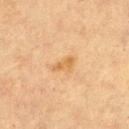Automated tile analysis of the lesion measured a footprint of about 3 mm², an outline eccentricity of about 0.9 (0 = round, 1 = elongated), and a symmetry-axis asymmetry near 0.4. The analysis additionally found a normalized lesion–skin contrast near 6.5. The analysis additionally found a border-irregularity index near 3.5/10 and peripheral color asymmetry of about 0.
A female subject aged 53 to 57.
The lesion's longest dimension is about 3 mm.
A close-up tile cropped from a whole-body skin photograph, about 15 mm across.
On the left thigh.
This is a cross-polarized tile.